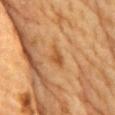Case summary:
* workup · total-body-photography surveillance lesion; no biopsy
* body site · the front of the torso
* illumination · cross-polarized illumination
* imaging modality · total-body-photography crop, ~15 mm field of view
* automated lesion analysis · a footprint of about 3 mm² and a symmetry-axis asymmetry near 0.45; a mean CIELAB color near L≈46 a*≈21 b*≈38, roughly 8 lightness units darker than nearby skin, and a lesion-to-skin contrast of about 6.5 (normalized; higher = more distinct); a classifier nevus-likeness of about 0/100 and a detector confidence of about 100 out of 100 that the crop contains a lesion
* patient · male, aged 83 or over
* size · about 2.5 mm